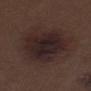  biopsy_status: not biopsied; imaged during a skin examination
  lesion_size:
    long_diameter_mm_approx: 9.5
  site: left thigh
  patient:
    sex: male
    age_approx: 70
  image:
    source: total-body photography crop
    field_of_view_mm: 15